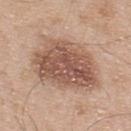Assessment:
Part of a total-body skin-imaging series; this lesion was reviewed on a skin check and was not flagged for biopsy.
Background:
The lesion-visualizer software estimated a nevus-likeness score of about 25/100 and a detector confidence of about 100 out of 100 that the crop contains a lesion. A male patient in their mid-50s. A lesion tile, about 15 mm wide, cut from a 3D total-body photograph. Located on the upper back. Imaged with white-light lighting. The lesion's longest dimension is about 8.5 mm.